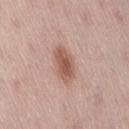No biopsy was performed on this lesion — it was imaged during a full skin examination and was not determined to be concerning.
This is a white-light tile.
From the lower back.
The lesion's longest dimension is about 4.5 mm.
The subject is a female approximately 65 years of age.
Cropped from a whole-body photographic skin survey; the tile spans about 15 mm.